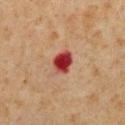Recorded during total-body skin imaging; not selected for excision or biopsy. The tile uses cross-polarized illumination. About 3.5 mm across. A male subject about 60 years old. This image is a 15 mm lesion crop taken from a total-body photograph. Located on the chest.